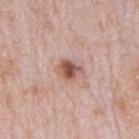Q: Patient demographics?
A: male, about 80 years old
Q: What kind of image is this?
A: total-body-photography crop, ~15 mm field of view
Q: Automated lesion metrics?
A: a border-irregularity rating of about 2/10 and radial color variation of about 1.5; a lesion-detection confidence of about 100/100
Q: Lesion size?
A: ~3 mm (longest diameter)
Q: How was the tile lit?
A: white-light illumination
Q: Lesion location?
A: the mid back